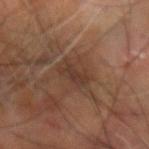Impression:
The lesion was photographed on a routine skin check and not biopsied; there is no pathology result.
Context:
From the right arm. The patient is a male approximately 60 years of age. The total-body-photography lesion software estimated a footprint of about 6.5 mm², an outline eccentricity of about 0.9 (0 = round, 1 = elongated), and two-axis asymmetry of about 0.25. And it measured a border-irregularity index near 3.5/10 and a within-lesion color-variation index near 2/10. Imaged with cross-polarized lighting. About 4 mm across. Cropped from a whole-body photographic skin survey; the tile spans about 15 mm.